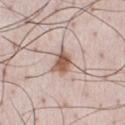Impression: The lesion was photographed on a routine skin check and not biopsied; there is no pathology result. Acquisition and patient details: A lesion tile, about 15 mm wide, cut from a 3D total-body photograph. Located on the front of the torso. A male subject roughly 35 years of age.